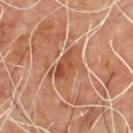biopsy status: catalogued during a skin exam; not biopsied
anatomic site: the upper back
image source: 15 mm crop, total-body photography
automated metrics: a lesion color around L≈48 a*≈26 b*≈33 in CIELAB, about 9 CIELAB-L* units darker than the surrounding skin, and a lesion-to-skin contrast of about 7 (normalized; higher = more distinct); a border-irregularity rating of about 3.5/10 and internal color variation of about 4 on a 0–10 scale; a nevus-likeness score of about 0/100 and a lesion-detection confidence of about 60/100
lesion size: ≈4.5 mm
tile lighting: cross-polarized
subject: male, about 60 years old